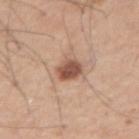The lesion was photographed on a routine skin check and not biopsied; there is no pathology result.
A male subject approximately 60 years of age.
An algorithmic analysis of the crop reported internal color variation of about 3 on a 0–10 scale.
The tile uses white-light illumination.
A 15 mm crop from a total-body photograph taken for skin-cancer surveillance.
From the arm.